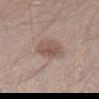biopsy_status: not biopsied; imaged during a skin examination
patient:
  sex: male
  age_approx: 30
lighting: white-light
lesion_size:
  long_diameter_mm_approx: 4.0
automated_metrics:
  area_mm2_approx: 9.5
  shape_asymmetry: 0.15
  vs_skin_contrast_norm: 6.5
image:
  source: total-body photography crop
  field_of_view_mm: 15
site: right thigh